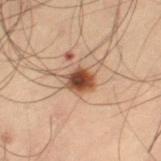On the left thigh. A region of skin cropped from a whole-body photographic capture, roughly 15 mm wide. A male subject, in their mid-50s. Measured at roughly 3 mm in maximum diameter.